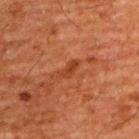Notes:
• follow-up: total-body-photography surveillance lesion; no biopsy
• location: the upper back
• acquisition: ~15 mm tile from a whole-body skin photo
• patient: male, aged around 60
• size: about 2.5 mm